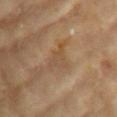No biopsy was performed on this lesion — it was imaged during a full skin examination and was not determined to be concerning. A female subject, aged around 75. Located on the left upper arm. An algorithmic analysis of the crop reported an area of roughly 4.5 mm² and a symmetry-axis asymmetry near 0.55. The analysis additionally found a mean CIELAB color near L≈49 a*≈17 b*≈32, about 6 CIELAB-L* units darker than the surrounding skin, and a lesion-to-skin contrast of about 4.5 (normalized; higher = more distinct). The analysis additionally found a border-irregularity index near 7/10, a within-lesion color-variation index near 2.5/10, and radial color variation of about 1. And it measured a classifier nevus-likeness of about 0/100 and a detector confidence of about 95 out of 100 that the crop contains a lesion. Longest diameter approximately 4 mm. The tile uses cross-polarized illumination. Cropped from a total-body skin-imaging series; the visible field is about 15 mm.